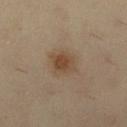Assessment: Part of a total-body skin-imaging series; this lesion was reviewed on a skin check and was not flagged for biopsy. Context: A female subject aged 38 to 42. From the right lower leg. The recorded lesion diameter is about 4 mm. A 15 mm close-up tile from a total-body photography series done for melanoma screening.